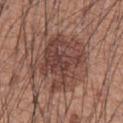notes = no biopsy performed (imaged during a skin exam)
lesion diameter = ≈7.5 mm
site = the front of the torso
tile lighting = white-light
patient = male, in their 60s
image = ~15 mm tile from a whole-body skin photo
image-analysis metrics = a color-variation rating of about 5/10 and a peripheral color-asymmetry measure near 2